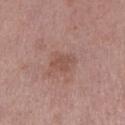Q: Is there a histopathology result?
A: imaged on a skin check; not biopsied
Q: Who is the patient?
A: female, roughly 40 years of age
Q: How large is the lesion?
A: about 3 mm
Q: What lighting was used for the tile?
A: white-light
Q: What kind of image is this?
A: total-body-photography crop, ~15 mm field of view
Q: What is the anatomic site?
A: the left lower leg